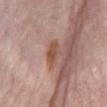workup: catalogued during a skin exam; not biopsied
subject: male, approximately 75 years of age
lighting: white-light
site: the back
acquisition: ~15 mm crop, total-body skin-cancer survey
automated metrics: a symmetry-axis asymmetry near 0.25; an average lesion color of about L≈52 a*≈21 b*≈29 (CIELAB), about 10 CIELAB-L* units darker than the surrounding skin, and a normalized border contrast of about 8; a nevus-likeness score of about 5/100